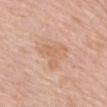Impression:
Recorded during total-body skin imaging; not selected for excision or biopsy.